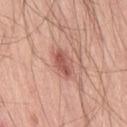Captured during whole-body skin photography for melanoma surveillance; the lesion was not biopsied.
The lesion-visualizer software estimated a lesion area of about 5.5 mm² and two-axis asymmetry of about 0.15. The analysis additionally found a lesion color around L≈55 a*≈27 b*≈27 in CIELAB and a lesion–skin lightness drop of about 12. It also reported a nevus-likeness score of about 70/100.
Captured under white-light illumination.
The recorded lesion diameter is about 3 mm.
A close-up tile cropped from a whole-body skin photograph, about 15 mm across.
The lesion is on the right thigh.
The subject is a male aged 53–57.